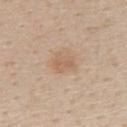Case summary:
* notes: total-body-photography surveillance lesion; no biopsy
* lighting: white-light illumination
* patient: female, aged around 45
* body site: the back
* acquisition: ~15 mm tile from a whole-body skin photo
* diameter: about 2.5 mm